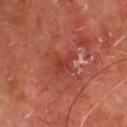| key | value |
|---|---|
| follow-up | catalogued during a skin exam; not biopsied |
| subject | male, aged 63 to 67 |
| body site | the chest |
| automated lesion analysis | a footprint of about 5 mm² and a symmetry-axis asymmetry near 0.4 |
| imaging modality | 15 mm crop, total-body photography |